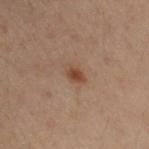| feature | finding |
|---|---|
| workup | no biopsy performed (imaged during a skin exam) |
| diameter | ~3 mm (longest diameter) |
| location | the left arm |
| imaging modality | ~15 mm crop, total-body skin-cancer survey |
| patient | female, in their mid- to late 50s |